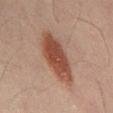Q: Is there a histopathology result?
A: no biopsy performed (imaged during a skin exam)
Q: Illumination type?
A: cross-polarized illumination
Q: What are the patient's age and sex?
A: male, roughly 50 years of age
Q: What is the imaging modality?
A: ~15 mm tile from a whole-body skin photo
Q: Lesion location?
A: the mid back
Q: What is the lesion's diameter?
A: about 7.5 mm
Q: What did automated image analysis measure?
A: roughly 12 lightness units darker than nearby skin and a normalized border contrast of about 10; a lesion-detection confidence of about 100/100